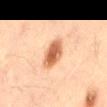{
  "biopsy_status": "not biopsied; imaged during a skin examination",
  "patient": {
    "sex": "male",
    "age_approx": 50
  },
  "lighting": "cross-polarized",
  "site": "mid back",
  "lesion_size": {
    "long_diameter_mm_approx": 4.0
  },
  "automated_metrics": {
    "area_mm2_approx": 8.5,
    "eccentricity": 0.8,
    "cielab_L": 58,
    "cielab_a": 24,
    "cielab_b": 35,
    "vs_skin_darker_L": 15.0,
    "vs_skin_contrast_norm": 9.5,
    "peripheral_color_asymmetry": 1.5,
    "lesion_detection_confidence_0_100": 100
  },
  "image": {
    "source": "total-body photography crop",
    "field_of_view_mm": 15
  }
}